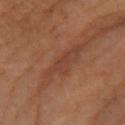Recorded during total-body skin imaging; not selected for excision or biopsy.
This is a cross-polarized tile.
The patient is a female aged around 55.
A roughly 15 mm field-of-view crop from a total-body skin photograph.
Located on the arm.